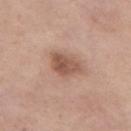Captured during whole-body skin photography for melanoma surveillance; the lesion was not biopsied.
An algorithmic analysis of the crop reported a footprint of about 8 mm². It also reported a border-irregularity rating of about 3/10 and internal color variation of about 4 on a 0–10 scale. And it measured a nevus-likeness score of about 65/100 and a lesion-detection confidence of about 100/100.
Captured under white-light illumination.
A female patient, about 55 years old.
On the left thigh.
A close-up tile cropped from a whole-body skin photograph, about 15 mm across.
Longest diameter approximately 4 mm.